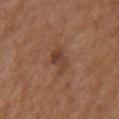biopsy_status: not biopsied; imaged during a skin examination
site: arm
image:
  source: total-body photography crop
  field_of_view_mm: 15
automated_metrics:
  area_mm2_approx: 5.0
  eccentricity: 0.75
lesion_size:
  long_diameter_mm_approx: 3.0
patient:
  sex: male
  age_approx: 75